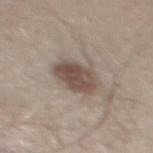Case summary:
* biopsy status: catalogued during a skin exam; not biopsied
* imaging modality: ~15 mm crop, total-body skin-cancer survey
* subject: male, in their 30s
* lesion diameter: ≈4 mm
* location: the mid back
* lighting: white-light